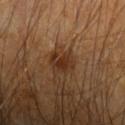Notes:
– workup · no biopsy performed (imaged during a skin exam)
– diameter · ~3 mm (longest diameter)
– subject · male, aged around 60
– site · the left forearm
– image source · 15 mm crop, total-body photography
– automated metrics · a lesion color around L≈29 a*≈18 b*≈28 in CIELAB and a lesion-to-skin contrast of about 8.5 (normalized; higher = more distinct); a border-irregularity index near 3.5/10 and peripheral color asymmetry of about 1; an automated nevus-likeness rating near 50 out of 100 and lesion-presence confidence of about 100/100
– illumination · cross-polarized